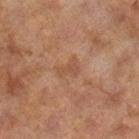  biopsy_status: not biopsied; imaged during a skin examination
  image:
    source: total-body photography crop
    field_of_view_mm: 15
  lesion_size:
    long_diameter_mm_approx: 2.5
  site: right lower leg
  patient:
    sex: female
    age_approx: 60
  lighting: cross-polarized